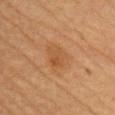Captured under cross-polarized illumination.
A close-up tile cropped from a whole-body skin photograph, about 15 mm across.
The subject is a female aged approximately 45.
The total-body-photography lesion software estimated a mean CIELAB color near L≈46 a*≈20 b*≈34, roughly 6 lightness units darker than nearby skin, and a normalized border contrast of about 5. The analysis additionally found border irregularity of about 3 on a 0–10 scale, a color-variation rating of about 3.5/10, and peripheral color asymmetry of about 1. The software also gave a lesion-detection confidence of about 100/100.
On the head or neck.
The recorded lesion diameter is about 4 mm.